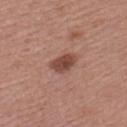The lesion was tiled from a total-body skin photograph and was not biopsied. A roughly 15 mm field-of-view crop from a total-body skin photograph. The lesion's longest dimension is about 3.5 mm. The lesion-visualizer software estimated a border-irregularity rating of about 2/10, internal color variation of about 2.5 on a 0–10 scale, and peripheral color asymmetry of about 0.5. The software also gave an automated nevus-likeness rating near 85 out of 100 and lesion-presence confidence of about 100/100. Imaged with white-light lighting. A female patient aged 48–52. The lesion is located on the left upper arm.